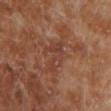Captured under cross-polarized illumination.
A lesion tile, about 15 mm wide, cut from a 3D total-body photograph.
An algorithmic analysis of the crop reported a shape eccentricity near 0.85.
Located on the left lower leg.
A male patient in their 70s.
The lesion's longest dimension is about 4.5 mm.